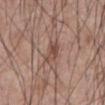{"biopsy_status": "not biopsied; imaged during a skin examination", "patient": {"sex": "male", "age_approx": 60}, "lesion_size": {"long_diameter_mm_approx": 3.0}, "image": {"source": "total-body photography crop", "field_of_view_mm": 15}, "site": "abdomen", "lighting": "white-light"}